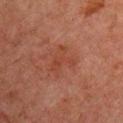Captured during whole-body skin photography for melanoma surveillance; the lesion was not biopsied.
The subject is a male approximately 60 years of age.
The lesion is located on the chest.
The tile uses cross-polarized illumination.
The lesion's longest dimension is about 3.5 mm.
This image is a 15 mm lesion crop taken from a total-body photograph.